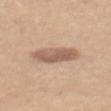Assessment: Captured during whole-body skin photography for melanoma surveillance; the lesion was not biopsied. Clinical summary: A female subject aged 53 to 57. The lesion is on the back. The recorded lesion diameter is about 4.5 mm. The total-body-photography lesion software estimated a lesion color around L≈59 a*≈19 b*≈27 in CIELAB and a normalized border contrast of about 7.5. Cropped from a whole-body photographic skin survey; the tile spans about 15 mm. Captured under white-light illumination.